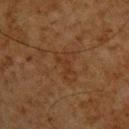<lesion>
  <biopsy_status>not biopsied; imaged during a skin examination</biopsy_status>
  <lighting>cross-polarized</lighting>
  <automated_metrics>
    <lesion_detection_confidence_0_100>100</lesion_detection_confidence_0_100>
  </automated_metrics>
  <site>head or neck</site>
  <image>
    <source>total-body photography crop</source>
    <field_of_view_mm>15</field_of_view_mm>
  </image>
  <lesion_size>
    <long_diameter_mm_approx>3.5</long_diameter_mm_approx>
  </lesion_size>
  <patient>
    <sex>male</sex>
    <age_approx>65</age_approx>
  </patient>
</lesion>